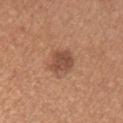Q: Is there a histopathology result?
A: no biopsy performed (imaged during a skin exam)